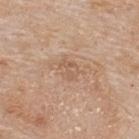Context: The total-body-photography lesion software estimated a lesion area of about 4 mm², an eccentricity of roughly 0.65, and two-axis asymmetry of about 0.35. And it measured an average lesion color of about L≈58 a*≈18 b*≈31 (CIELAB), about 6 CIELAB-L* units darker than the surrounding skin, and a normalized border contrast of about 4.5. The analysis additionally found a border-irregularity rating of about 4/10, a color-variation rating of about 2.5/10, and peripheral color asymmetry of about 1. The analysis additionally found a lesion-detection confidence of about 100/100. A region of skin cropped from a whole-body photographic capture, roughly 15 mm wide. The lesion is located on the upper back. About 2.5 mm across. The tile uses white-light illumination. The subject is a male about 55 years old.